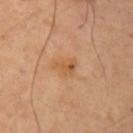No biopsy was performed on this lesion — it was imaged during a full skin examination and was not determined to be concerning.
Captured under cross-polarized illumination.
A female subject, aged around 45.
Longest diameter approximately 2.5 mm.
From the left upper arm.
A region of skin cropped from a whole-body photographic capture, roughly 15 mm wide.